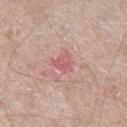imaging modality=~15 mm tile from a whole-body skin photo; anatomic site=the right thigh; diameter=about 3 mm; subject=male, in their mid-60s; tile lighting=white-light illumination.